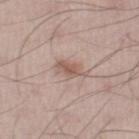Recorded during total-body skin imaging; not selected for excision or biopsy. A 15 mm crop from a total-body photograph taken for skin-cancer surveillance. The patient is a male in their mid-50s. On the leg.